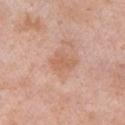Notes:
– workup · imaged on a skin check; not biopsied
– body site · the left upper arm
– subject · male, about 55 years old
– image source · ~15 mm tile from a whole-body skin photo